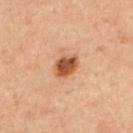{
  "biopsy_status": "not biopsied; imaged during a skin examination",
  "site": "upper back",
  "patient": {
    "sex": "male",
    "age_approx": 55
  },
  "lesion_size": {
    "long_diameter_mm_approx": 3.0
  },
  "image": {
    "source": "total-body photography crop",
    "field_of_view_mm": 15
  },
  "automated_metrics": {
    "cielab_L": 54,
    "cielab_a": 26,
    "cielab_b": 38,
    "vs_skin_darker_L": 16.0,
    "vs_skin_contrast_norm": 10.5,
    "border_irregularity_0_10": 1.5,
    "color_variation_0_10": 4.5,
    "peripheral_color_asymmetry": 1.5
  }
}